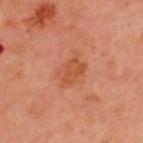The lesion was photographed on a routine skin check and not biopsied; there is no pathology result.
The tile uses cross-polarized illumination.
The subject is a male approximately 50 years of age.
A region of skin cropped from a whole-body photographic capture, roughly 15 mm wide.
The lesion's longest dimension is about 3.5 mm.
On the upper back.